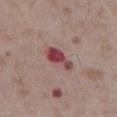Imaged during a routine full-body skin examination; the lesion was not biopsied and no histopathology is available. Located on the abdomen. The lesion's longest dimension is about 4 mm. Captured under white-light illumination. The lesion-visualizer software estimated a lesion area of about 6.5 mm², an outline eccentricity of about 0.85 (0 = round, 1 = elongated), and two-axis asymmetry of about 0.35. It also reported border irregularity of about 3.5 on a 0–10 scale, a color-variation rating of about 4/10, and peripheral color asymmetry of about 1. It also reported a classifier nevus-likeness of about 0/100 and a detector confidence of about 100 out of 100 that the crop contains a lesion. The subject is a male approximately 75 years of age. A close-up tile cropped from a whole-body skin photograph, about 15 mm across.